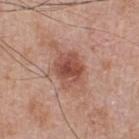biopsy_status: not biopsied; imaged during a skin examination
lighting: white-light
site: upper back
patient:
  sex: male
  age_approx: 65
image:
  source: total-body photography crop
  field_of_view_mm: 15